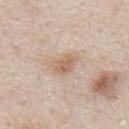{
  "biopsy_status": "not biopsied; imaged during a skin examination"
}